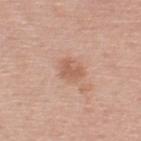Notes:
• biopsy status · no biopsy performed (imaged during a skin exam)
• acquisition · ~15 mm crop, total-body skin-cancer survey
• subject · male, aged 63–67
• automated lesion analysis · an automated nevus-likeness rating near 15 out of 100
• location · the upper back
• diameter · ≈3 mm
• lighting · white-light